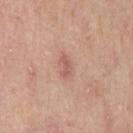Recorded during total-body skin imaging; not selected for excision or biopsy. A 15 mm close-up extracted from a 3D total-body photography capture. A female subject, approximately 50 years of age. From the right thigh.